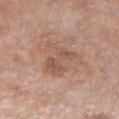workup=imaged on a skin check; not biopsied | lighting=white-light | subject=male, aged approximately 60 | site=the chest | TBP lesion metrics=a footprint of about 14 mm² and a symmetry-axis asymmetry near 0.45; a nevus-likeness score of about 0/100 and a lesion-detection confidence of about 100/100 | imaging modality=15 mm crop, total-body photography.